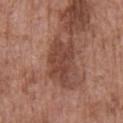This lesion was catalogued during total-body skin photography and was not selected for biopsy.
The recorded lesion diameter is about 6 mm.
On the front of the torso.
This is a white-light tile.
The total-body-photography lesion software estimated a mean CIELAB color near L≈44 a*≈22 b*≈27, roughly 10 lightness units darker than nearby skin, and a lesion-to-skin contrast of about 8 (normalized; higher = more distinct). The software also gave a border-irregularity rating of about 4.5/10, a color-variation rating of about 3/10, and peripheral color asymmetry of about 1.
A male subject, roughly 75 years of age.
A lesion tile, about 15 mm wide, cut from a 3D total-body photograph.